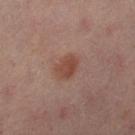Q: Is there a histopathology result?
A: catalogued during a skin exam; not biopsied
Q: What is the imaging modality?
A: ~15 mm tile from a whole-body skin photo
Q: What lighting was used for the tile?
A: cross-polarized
Q: What are the patient's age and sex?
A: female, approximately 50 years of age
Q: What did automated image analysis measure?
A: a footprint of about 6 mm² and a shape eccentricity near 0.7; a nevus-likeness score of about 95/100
Q: What is the lesion's diameter?
A: ~3 mm (longest diameter)
Q: What is the anatomic site?
A: the left thigh